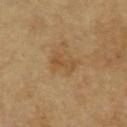Clinical impression: Imaged during a routine full-body skin examination; the lesion was not biopsied and no histopathology is available. Clinical summary: The subject is a male aged approximately 65. Approximately 3 mm at its widest. Cropped from a whole-body photographic skin survey; the tile spans about 15 mm. An algorithmic analysis of the crop reported a lesion color around L≈50 a*≈18 b*≈36 in CIELAB, roughly 6 lightness units darker than nearby skin, and a normalized border contrast of about 5. The analysis additionally found a within-lesion color-variation index near 3/10. This is a cross-polarized tile. Located on the chest.